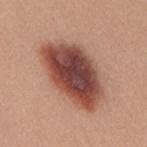Image and clinical context:
This is a white-light tile. Cropped from a whole-body photographic skin survey; the tile spans about 15 mm. A female subject, aged 33 to 37. Located on the upper back. The recorded lesion diameter is about 9 mm.